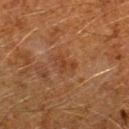Notes:
• follow-up · imaged on a skin check; not biopsied
• automated metrics · a footprint of about 4 mm² and a symmetry-axis asymmetry near 0.4; a border-irregularity index near 4/10, a color-variation rating of about 2/10, and a peripheral color-asymmetry measure near 0.5; a classifier nevus-likeness of about 0/100 and a lesion-detection confidence of about 100/100
• patient · male, aged 58 to 62
• lesion diameter · about 3 mm
• acquisition · ~15 mm tile from a whole-body skin photo
• tile lighting · cross-polarized illumination
• anatomic site · the left forearm